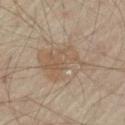workup: no biopsy performed (imaged during a skin exam) | subject: about 55 years old | body site: the left thigh | image: 15 mm crop, total-body photography.